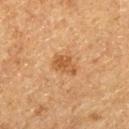Assessment: Captured during whole-body skin photography for melanoma surveillance; the lesion was not biopsied. Acquisition and patient details: The recorded lesion diameter is about 3 mm. The tile uses cross-polarized illumination. A male patient, aged 73–77. Located on the left thigh. A roughly 15 mm field-of-view crop from a total-body skin photograph.